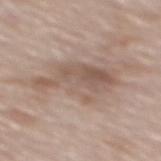Clinical impression:
No biopsy was performed on this lesion — it was imaged during a full skin examination and was not determined to be concerning.
Acquisition and patient details:
A region of skin cropped from a whole-body photographic capture, roughly 15 mm wide. A male subject roughly 75 years of age. On the mid back.